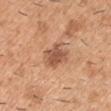Q: Was this lesion biopsied?
A: no biopsy performed (imaged during a skin exam)
Q: Patient demographics?
A: male, in their 40s
Q: What is the anatomic site?
A: the right upper arm
Q: What lighting was used for the tile?
A: white-light
Q: How large is the lesion?
A: ≈4 mm
Q: What is the imaging modality?
A: 15 mm crop, total-body photography